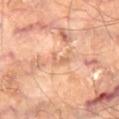Imaged during a routine full-body skin examination; the lesion was not biopsied and no histopathology is available.
The tile uses cross-polarized illumination.
Automated tile analysis of the lesion measured a mean CIELAB color near L≈64 a*≈23 b*≈34 and roughly 8 lightness units darker than nearby skin. The analysis additionally found a border-irregularity index near 7.5/10, a color-variation rating of about 0/10, and peripheral color asymmetry of about 0.
The subject is a male aged 68 to 72.
From the right thigh.
A 15 mm close-up tile from a total-body photography series done for melanoma screening.